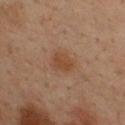{
  "biopsy_status": "not biopsied; imaged during a skin examination",
  "image": {
    "source": "total-body photography crop",
    "field_of_view_mm": 15
  },
  "lighting": "cross-polarized",
  "site": "upper back",
  "lesion_size": {
    "long_diameter_mm_approx": 3.0
  },
  "patient": {
    "sex": "male",
    "age_approx": 35
  }
}